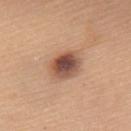automated_metrics:
  area_mm2_approx: 9.5
  eccentricity: 0.7
  shape_asymmetry: 0.15
  cielab_L: 51
  cielab_a: 22
  cielab_b: 28
  vs_skin_contrast_norm: 11.0
  border_irregularity_0_10: 1.5
  color_variation_0_10: 7.5
patient:
  sex: female
  age_approx: 65
site: upper back
lesion_size:
  long_diameter_mm_approx: 4.0
image:
  source: total-body photography crop
  field_of_view_mm: 15